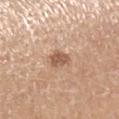  biopsy_status: not biopsied; imaged during a skin examination
  site: right lower leg
  lesion_size:
    long_diameter_mm_approx: 3.0
  patient:
    sex: male
    age_approx: 40
  automated_metrics:
    cielab_L: 56
    cielab_a: 20
    cielab_b: 30
    vs_skin_darker_L: 12.0
    vs_skin_contrast_norm: 8.0
    nevus_likeness_0_100: 10
  image:
    source: total-body photography crop
    field_of_view_mm: 15
  lighting: white-light